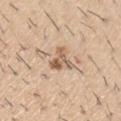Imaged during a routine full-body skin examination; the lesion was not biopsied and no histopathology is available. The subject is a male in their 60s. From the arm. A 15 mm close-up extracted from a 3D total-body photography capture.